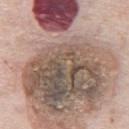Findings:
- image · ~15 mm crop, total-body skin-cancer survey
- lesion diameter · ≈17.5 mm
- automated lesion analysis · an average lesion color of about L≈53 a*≈17 b*≈22 (CIELAB) and a normalized lesion–skin contrast near 12; an automated nevus-likeness rating near 0 out of 100 and a detector confidence of about 100 out of 100 that the crop contains a lesion
- subject · male, approximately 75 years of age
- body site · the abdomen